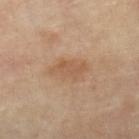follow-up: no biopsy performed (imaged during a skin exam)
subject: female, approximately 65 years of age
body site: the leg
tile lighting: cross-polarized
diameter: about 4.5 mm
image source: ~15 mm crop, total-body skin-cancer survey
automated metrics: a nevus-likeness score of about 0/100 and a detector confidence of about 100 out of 100 that the crop contains a lesion